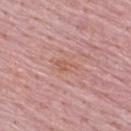notes: imaged on a skin check; not biopsied
lesion diameter: ≈3 mm
subject: male, about 65 years old
location: the upper back
automated lesion analysis: a mean CIELAB color near L≈58 a*≈24 b*≈28, about 6 CIELAB-L* units darker than the surrounding skin, and a lesion-to-skin contrast of about 5.5 (normalized; higher = more distinct); a border-irregularity rating of about 4.5/10, a color-variation rating of about 1/10, and radial color variation of about 0.5
image: 15 mm crop, total-body photography
lighting: white-light illumination